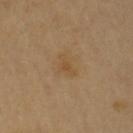Findings:
* biopsy status: total-body-photography surveillance lesion; no biopsy